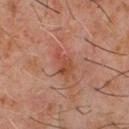Impression:
No biopsy was performed on this lesion — it was imaged during a full skin examination and was not determined to be concerning.
Image and clinical context:
The subject is a male in their 60s. A roughly 15 mm field-of-view crop from a total-body skin photograph. From the chest.